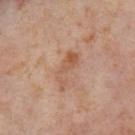Captured during whole-body skin photography for melanoma surveillance; the lesion was not biopsied. The lesion is on the right thigh. A 15 mm close-up extracted from a 3D total-body photography capture. A female patient, approximately 55 years of age. Captured under cross-polarized illumination. Longest diameter approximately 5 mm.